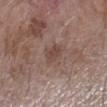{"biopsy_status": "not biopsied; imaged during a skin examination", "automated_metrics": {"eccentricity": 0.85, "shape_asymmetry": 0.25, "nevus_likeness_0_100": 0, "lesion_detection_confidence_0_100": 100}, "lesion_size": {"long_diameter_mm_approx": 3.5}, "site": "left forearm", "lighting": "white-light", "patient": {"sex": "male", "age_approx": 60}, "image": {"source": "total-body photography crop", "field_of_view_mm": 15}}